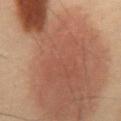Assessment: Recorded during total-body skin imaging; not selected for excision or biopsy. Background: On the abdomen. Captured under cross-polarized illumination. The total-body-photography lesion software estimated a shape eccentricity near 0.9 and a shape-asymmetry score of about 0.4 (0 = symmetric). And it measured a lesion color around L≈41 a*≈18 b*≈25 in CIELAB, roughly 11 lightness units darker than nearby skin, and a normalized border contrast of about 9. Longest diameter approximately 21 mm. The patient is a male aged around 55. A 15 mm close-up extracted from a 3D total-body photography capture.